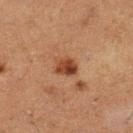Q: Was a biopsy performed?
A: imaged on a skin check; not biopsied
Q: Patient demographics?
A: female, in their 50s
Q: What did automated image analysis measure?
A: an outline eccentricity of about 0.55 (0 = round, 1 = elongated) and a shape-asymmetry score of about 0.2 (0 = symmetric); a lesion color around L≈36 a*≈21 b*≈29 in CIELAB, roughly 10 lightness units darker than nearby skin, and a normalized border contrast of about 9
Q: Where on the body is the lesion?
A: the left lower leg
Q: How large is the lesion?
A: ~3 mm (longest diameter)
Q: Illumination type?
A: cross-polarized
Q: What kind of image is this?
A: ~15 mm tile from a whole-body skin photo